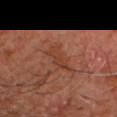Clinical summary:
The patient is a male in their 60s. The recorded lesion diameter is about 4.5 mm. Located on the head or neck. The lesion-visualizer software estimated an eccentricity of roughly 0.95 and a symmetry-axis asymmetry near 0.4. Cropped from a whole-body photographic skin survey; the tile spans about 15 mm. The tile uses cross-polarized illumination.